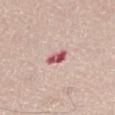Impression:
The lesion was tiled from a total-body skin photograph and was not biopsied.
Image and clinical context:
The lesion-visualizer software estimated a lesion area of about 3.5 mm² and a shape-asymmetry score of about 0.3 (0 = symmetric). And it measured border irregularity of about 3 on a 0–10 scale, a within-lesion color-variation index near 8/10, and a peripheral color-asymmetry measure near 3. About 2.5 mm across. On the right thigh. The subject is a male approximately 65 years of age. A lesion tile, about 15 mm wide, cut from a 3D total-body photograph.